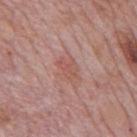A male patient, aged 73 to 77. Cropped from a whole-body photographic skin survey; the tile spans about 15 mm. The lesion-visualizer software estimated a lesion–skin lightness drop of about 7. It also reported a within-lesion color-variation index near 2.5/10 and a peripheral color-asymmetry measure near 1. The analysis additionally found a nevus-likeness score of about 5/100 and a lesion-detection confidence of about 100/100. The lesion's longest dimension is about 3 mm. The lesion is on the back. The tile uses white-light illumination.